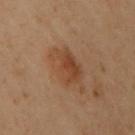Notes:
– workup · total-body-photography surveillance lesion; no biopsy
– anatomic site · the upper back
– automated lesion analysis · an area of roughly 11 mm², an outline eccentricity of about 0.6 (0 = round, 1 = elongated), and two-axis asymmetry of about 0.25; a mean CIELAB color near L≈38 a*≈18 b*≈28, roughly 7 lightness units darker than nearby skin, and a lesion-to-skin contrast of about 6.5 (normalized; higher = more distinct); a within-lesion color-variation index near 4/10 and peripheral color asymmetry of about 1.5; lesion-presence confidence of about 100/100
– subject · female, roughly 60 years of age
– image source · ~15 mm crop, total-body skin-cancer survey
– tile lighting · cross-polarized illumination
– diameter · ~4 mm (longest diameter)